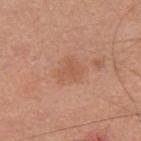{"lighting": "white-light", "lesion_size": {"long_diameter_mm_approx": 3.5}, "automated_metrics": {"area_mm2_approx": 7.5, "eccentricity": 0.45, "shape_asymmetry": 0.3, "vs_skin_darker_L": 6.0, "vs_skin_contrast_norm": 4.5, "border_irregularity_0_10": 3.0, "color_variation_0_10": 2.0, "peripheral_color_asymmetry": 0.5, "lesion_detection_confidence_0_100": 100}, "site": "upper back", "image": {"source": "total-body photography crop", "field_of_view_mm": 15}, "patient": {"sex": "male", "age_approx": 30}}